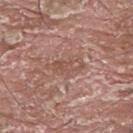notes — catalogued during a skin exam; not biopsied
location — the upper back
illumination — white-light illumination
subject — male, approximately 40 years of age
acquisition — ~15 mm crop, total-body skin-cancer survey